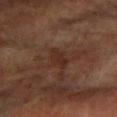| field | value |
|---|---|
| workup | no biopsy performed (imaged during a skin exam) |
| anatomic site | the left forearm |
| acquisition | ~15 mm tile from a whole-body skin photo |
| subject | female, about 60 years old |